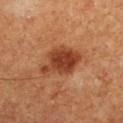Background: The lesion's longest dimension is about 5.5 mm. On the right lower leg. Captured under cross-polarized illumination. The patient is a male approximately 60 years of age. A 15 mm close-up extracted from a 3D total-body photography capture.